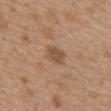  biopsy_status: not biopsied; imaged during a skin examination
  site: upper back
  patient:
    sex: female
    age_approx: 25
  lighting: white-light
  image:
    source: total-body photography crop
    field_of_view_mm: 15
  lesion_size:
    long_diameter_mm_approx: 2.5